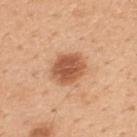  biopsy_status: not biopsied; imaged during a skin examination
  patient:
    sex: male
    age_approx: 50
  lighting: white-light
  site: upper back
  image:
    source: total-body photography crop
    field_of_view_mm: 15